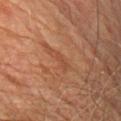Imaged during a routine full-body skin examination; the lesion was not biopsied and no histopathology is available.
The lesion-visualizer software estimated a mean CIELAB color near L≈39 a*≈21 b*≈27, a lesion–skin lightness drop of about 5, and a lesion-to-skin contrast of about 4.5 (normalized; higher = more distinct). It also reported a border-irregularity index near 5/10, a within-lesion color-variation index near 0/10, and radial color variation of about 0.
From the right upper arm.
The recorded lesion diameter is about 3 mm.
The patient is a male aged 73 to 77.
A 15 mm close-up extracted from a 3D total-body photography capture.
This is a cross-polarized tile.